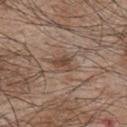{"biopsy_status": "not biopsied; imaged during a skin examination", "lesion_size": {"long_diameter_mm_approx": 3.0}, "site": "upper back", "image": {"source": "total-body photography crop", "field_of_view_mm": 15}, "lighting": "white-light", "patient": {"sex": "male", "age_approx": 45}}